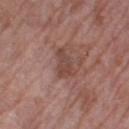Impression:
This lesion was catalogued during total-body skin photography and was not selected for biopsy.
Context:
A 15 mm close-up extracted from a 3D total-body photography capture. Located on the left thigh. Imaged with white-light lighting. A female subject aged approximately 70. Longest diameter approximately 3.5 mm.